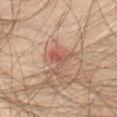The lesion was tiled from a total-body skin photograph and was not biopsied. Cropped from a whole-body photographic skin survey; the tile spans about 15 mm. A male subject, roughly 70 years of age. This is a cross-polarized tile. From the abdomen. Approximately 2.5 mm at its widest.